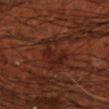{
  "biopsy_status": "not biopsied; imaged during a skin examination",
  "automated_metrics": {
    "cielab_L": 23,
    "cielab_a": 22,
    "cielab_b": 24,
    "vs_skin_darker_L": 6.0,
    "vs_skin_contrast_norm": 6.5,
    "nevus_likeness_0_100": 0,
    "lesion_detection_confidence_0_100": 60
  },
  "patient": {
    "sex": "male",
    "age_approx": 70
  },
  "image": {
    "source": "total-body photography crop",
    "field_of_view_mm": 15
  },
  "lighting": "cross-polarized",
  "lesion_size": {
    "long_diameter_mm_approx": 5.0
  },
  "site": "left forearm"
}